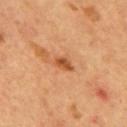Impression:
Captured during whole-body skin photography for melanoma surveillance; the lesion was not biopsied.
Image and clinical context:
Cropped from a whole-body photographic skin survey; the tile spans about 15 mm. On the mid back. A male patient in their mid-50s.